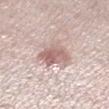notes: total-body-photography surveillance lesion; no biopsy
imaging modality: ~15 mm crop, total-body skin-cancer survey
lesion diameter: about 4 mm
illumination: white-light
subject: female, aged around 45
anatomic site: the left lower leg